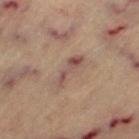Clinical impression:
The lesion was photographed on a routine skin check and not biopsied; there is no pathology result.
Acquisition and patient details:
A roughly 15 mm field-of-view crop from a total-body skin photograph. An algorithmic analysis of the crop reported a lesion area of about 5.5 mm², an eccentricity of roughly 0.95, and a shape-asymmetry score of about 0.45 (0 = symmetric). Located on the left thigh. Measured at roughly 4.5 mm in maximum diameter. A female subject, aged around 65. Captured under cross-polarized illumination.